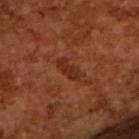Q: Is there a histopathology result?
A: no biopsy performed (imaged during a skin exam)
Q: What is the imaging modality?
A: ~15 mm crop, total-body skin-cancer survey
Q: What is the lesion's diameter?
A: ≈3.5 mm
Q: Who is the patient?
A: male, aged 63–67
Q: Automated lesion metrics?
A: a lesion color around L≈30 a*≈26 b*≈31 in CIELAB, roughly 7 lightness units darker than nearby skin, and a normalized border contrast of about 7; a color-variation rating of about 1.5/10 and peripheral color asymmetry of about 0.5; a nevus-likeness score of about 0/100 and a detector confidence of about 100 out of 100 that the crop contains a lesion
Q: How was the tile lit?
A: cross-polarized illumination